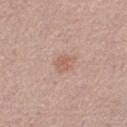* follow-up · imaged on a skin check; not biopsied
* illumination · white-light
* site · the right thigh
* image-analysis metrics · a symmetry-axis asymmetry near 0.35; a nevus-likeness score of about 10/100 and lesion-presence confidence of about 100/100
* size · about 2.5 mm
* subject · female, aged around 70
* image · total-body-photography crop, ~15 mm field of view